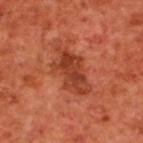Recorded during total-body skin imaging; not selected for excision or biopsy.
A 15 mm close-up extracted from a 3D total-body photography capture.
Imaged with cross-polarized lighting.
The total-body-photography lesion software estimated an average lesion color of about L≈40 a*≈30 b*≈35 (CIELAB) and roughly 10 lightness units darker than nearby skin. And it measured a border-irregularity index near 4/10 and a color-variation rating of about 4.5/10. The software also gave a classifier nevus-likeness of about 5/100 and a detector confidence of about 100 out of 100 that the crop contains a lesion.
The lesion is on the upper back.
About 6 mm across.
The subject is a male aged around 70.